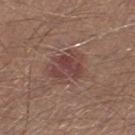Findings:
• notes · total-body-photography surveillance lesion; no biopsy
• lesion diameter · about 3.5 mm
• image · ~15 mm crop, total-body skin-cancer survey
• patient · male, aged 38–42
• lighting · white-light illumination
• body site · the right lower leg